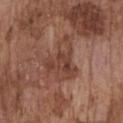Recorded during total-body skin imaging; not selected for excision or biopsy.
A close-up tile cropped from a whole-body skin photograph, about 15 mm across.
The subject is a male in their mid-70s.
From the front of the torso.
Imaged with white-light lighting.